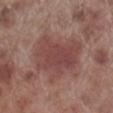Imaged during a routine full-body skin examination; the lesion was not biopsied and no histopathology is available. Cropped from a total-body skin-imaging series; the visible field is about 15 mm. Imaged with white-light lighting. The lesion's longest dimension is about 6.5 mm. The lesion is located on the right lower leg. Automated tile analysis of the lesion measured a border-irregularity index near 4/10 and peripheral color asymmetry of about 1. A male subject aged 68 to 72.